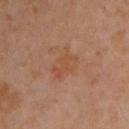Impression: Recorded during total-body skin imaging; not selected for excision or biopsy. Clinical summary: Approximately 4 mm at its widest. The subject is a male aged approximately 40. A close-up tile cropped from a whole-body skin photograph, about 15 mm across. From the front of the torso.